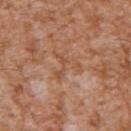Imaged during a routine full-body skin examination; the lesion was not biopsied and no histopathology is available. A male subject aged approximately 45. From the left upper arm. A 15 mm crop from a total-body photograph taken for skin-cancer surveillance. This is a white-light tile.